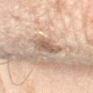Q: What are the patient's age and sex?
A: male, aged around 45
Q: How large is the lesion?
A: ≈3.5 mm
Q: Illumination type?
A: cross-polarized
Q: What is the anatomic site?
A: the right forearm
Q: What kind of image is this?
A: 15 mm crop, total-body photography